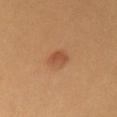  lesion_size:
    long_diameter_mm_approx: 3.0
  image:
    source: total-body photography crop
    field_of_view_mm: 15
  patient:
    sex: female
    age_approx: 50
  automated_metrics:
    eccentricity: 0.75
    shape_asymmetry: 0.2
    border_irregularity_0_10: 2.0
    nevus_likeness_0_100: 75
    lesion_detection_confidence_0_100: 100
  lighting: cross-polarized